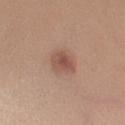- follow-up: imaged on a skin check; not biopsied
- imaging modality: total-body-photography crop, ~15 mm field of view
- site: the right upper arm
- subject: female, aged 53 to 57